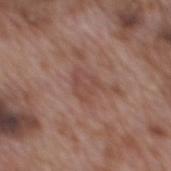| key | value |
|---|---|
| follow-up | no biopsy performed (imaged during a skin exam) |
| size | ~3 mm (longest diameter) |
| anatomic site | the mid back |
| image-analysis metrics | a footprint of about 6 mm², an outline eccentricity of about 0.55 (0 = round, 1 = elongated), and a symmetry-axis asymmetry near 0.3; about 6 CIELAB-L* units darker than the surrounding skin and a normalized border contrast of about 5; a border-irregularity rating of about 3.5/10; an automated nevus-likeness rating near 0 out of 100 and lesion-presence confidence of about 100/100 |
| illumination | white-light |
| image source | total-body-photography crop, ~15 mm field of view |
| patient | male, in their 70s |